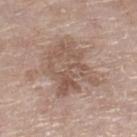Recorded during total-body skin imaging; not selected for excision or biopsy. Cropped from a whole-body photographic skin survey; the tile spans about 15 mm. About 7 mm across. The subject is a female aged 73–77. The tile uses white-light illumination. On the right lower leg.